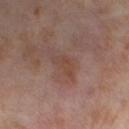The patient is a female aged around 55.
The total-body-photography lesion software estimated border irregularity of about 5 on a 0–10 scale, a color-variation rating of about 2/10, and radial color variation of about 0.5. And it measured a nevus-likeness score of about 0/100.
This is a cross-polarized tile.
The lesion's longest dimension is about 3.5 mm.
The lesion is located on the right thigh.
This image is a 15 mm lesion crop taken from a total-body photograph.